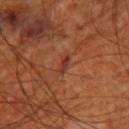Case summary:
- subject: male, aged around 70
- automated lesion analysis: a border-irregularity index near 4.5/10, internal color variation of about 0 on a 0–10 scale, and peripheral color asymmetry of about 0; a detector confidence of about 75 out of 100 that the crop contains a lesion
- tile lighting: cross-polarized illumination
- lesion diameter: ≈2.5 mm
- body site: the right thigh
- acquisition: ~15 mm crop, total-body skin-cancer survey